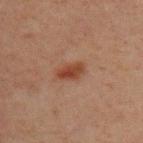{"site": "upper back", "lighting": "cross-polarized", "patient": {"sex": "male", "age_approx": 30}, "lesion_size": {"long_diameter_mm_approx": 3.0}, "image": {"source": "total-body photography crop", "field_of_view_mm": 15}}